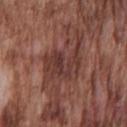follow-up = catalogued during a skin exam; not biopsied
subject = male, in their mid-70s
site = the chest
tile lighting = white-light illumination
size = ≈6.5 mm
image source = ~15 mm crop, total-body skin-cancer survey
image-analysis metrics = a lesion color around L≈37 a*≈22 b*≈23 in CIELAB, roughly 9 lightness units darker than nearby skin, and a normalized border contrast of about 7.5; a classifier nevus-likeness of about 0/100 and lesion-presence confidence of about 60/100